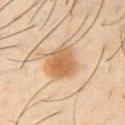lesion size: ≈4.5 mm | automated metrics: an eccentricity of roughly 0.65 and a shape-asymmetry score of about 0.2 (0 = symmetric); a border-irregularity index near 2/10, a within-lesion color-variation index near 4/10, and radial color variation of about 1.5 | site: the right upper arm | acquisition: 15 mm crop, total-body photography | subject: male, in their 40s | lighting: cross-polarized illumination.